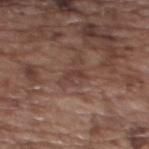<case>
<biopsy_status>not biopsied; imaged during a skin examination</biopsy_status>
<lighting>white-light</lighting>
<image>
  <source>total-body photography crop</source>
  <field_of_view_mm>15</field_of_view_mm>
</image>
<site>upper back</site>
<patient>
  <sex>female</sex>
  <age_approx>75</age_approx>
</patient>
<lesion_size>
  <long_diameter_mm_approx>2.5</long_diameter_mm_approx>
</lesion_size>
</case>